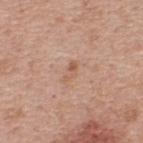notes: no biopsy performed (imaged during a skin exam)
size: about 2.5 mm
site: the back
automated lesion analysis: a mean CIELAB color near L≈58 a*≈20 b*≈31 and a lesion-to-skin contrast of about 6 (normalized; higher = more distinct); a color-variation rating of about 0/10; an automated nevus-likeness rating near 5 out of 100 and lesion-presence confidence of about 100/100
patient: male, aged 38 to 42
illumination: white-light
image source: 15 mm crop, total-body photography